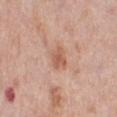Q: Is there a histopathology result?
A: imaged on a skin check; not biopsied
Q: What kind of image is this?
A: 15 mm crop, total-body photography
Q: What lighting was used for the tile?
A: white-light
Q: What is the lesion's diameter?
A: ~3 mm (longest diameter)
Q: What are the patient's age and sex?
A: female, roughly 65 years of age
Q: Lesion location?
A: the leg